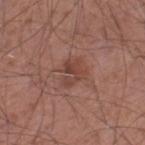Clinical impression: Captured during whole-body skin photography for melanoma surveillance; the lesion was not biopsied. Acquisition and patient details: Located on the left thigh. A male patient in their mid- to late 50s. A 15 mm crop from a total-body photograph taken for skin-cancer surveillance.